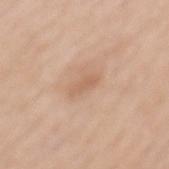Notes:
* follow-up — no biopsy performed (imaged during a skin exam)
* subject — female, in their mid-60s
* tile lighting — white-light
* anatomic site — the mid back
* imaging modality — total-body-photography crop, ~15 mm field of view
* automated metrics — a footprint of about 3.5 mm² and a symmetry-axis asymmetry near 0.25; an average lesion color of about L≈62 a*≈19 b*≈32 (CIELAB), a lesion–skin lightness drop of about 7, and a normalized lesion–skin contrast near 5
* lesion size — ~3 mm (longest diameter)